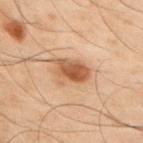notes: no biopsy performed (imaged during a skin exam) | image source: total-body-photography crop, ~15 mm field of view | site: the right upper arm | lighting: cross-polarized | patient: male, aged 48 to 52.